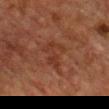Part of a total-body skin-imaging series; this lesion was reviewed on a skin check and was not flagged for biopsy. A close-up tile cropped from a whole-body skin photograph, about 15 mm across. Imaged with cross-polarized lighting. From the chest. About 4.5 mm across. A male subject aged around 70.